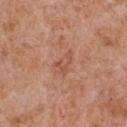biopsy status: no biopsy performed (imaged during a skin exam)
subject: male, roughly 65 years of age
automated metrics: an area of roughly 4 mm², an eccentricity of roughly 0.8, and a shape-asymmetry score of about 0.4 (0 = symmetric); a lesion color around L≈53 a*≈25 b*≈32 in CIELAB and a normalized border contrast of about 5; an automated nevus-likeness rating near 0 out of 100
image: ~15 mm crop, total-body skin-cancer survey
illumination: white-light illumination
anatomic site: the chest